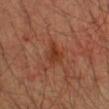Q: Was a biopsy performed?
A: catalogued during a skin exam; not biopsied
Q: What kind of image is this?
A: 15 mm crop, total-body photography
Q: What is the lesion's diameter?
A: about 3 mm
Q: Where on the body is the lesion?
A: the right forearm
Q: How was the tile lit?
A: cross-polarized illumination
Q: Who is the patient?
A: male, roughly 35 years of age